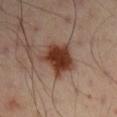- notes — total-body-photography surveillance lesion; no biopsy
- diameter — ≈5 mm
- body site — the arm
- patient — male, about 50 years old
- illumination — cross-polarized illumination
- imaging modality — total-body-photography crop, ~15 mm field of view